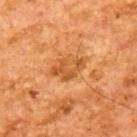No biopsy was performed on this lesion — it was imaged during a full skin examination and was not determined to be concerning.
A male subject aged 63 to 67.
The tile uses cross-polarized illumination.
About 4 mm across.
Automated tile analysis of the lesion measured a mean CIELAB color near L≈55 a*≈27 b*≈45 and about 10 CIELAB-L* units darker than the surrounding skin. And it measured lesion-presence confidence of about 100/100.
This image is a 15 mm lesion crop taken from a total-body photograph.